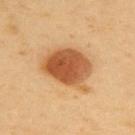  biopsy_status: not biopsied; imaged during a skin examination
  patient:
    sex: female
    age_approx: 50
  image:
    source: total-body photography crop
    field_of_view_mm: 15
  site: upper back